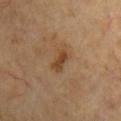{"biopsy_status": "not biopsied; imaged during a skin examination", "patient": {"age_approx": 65}, "lighting": "cross-polarized", "site": "arm", "image": {"source": "total-body photography crop", "field_of_view_mm": 15}, "automated_metrics": {"area_mm2_approx": 5.5, "eccentricity": 0.9, "shape_asymmetry": 0.3, "cielab_L": 44, "cielab_a": 18, "cielab_b": 33, "vs_skin_contrast_norm": 7.0, "color_variation_0_10": 3.0, "nevus_likeness_0_100": 25, "lesion_detection_confidence_0_100": 100}}